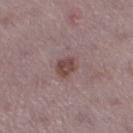| field | value |
|---|---|
| workup | no biopsy performed (imaged during a skin exam) |
| patient | female, aged approximately 50 |
| tile lighting | white-light illumination |
| acquisition | ~15 mm tile from a whole-body skin photo |
| lesion size | ~3 mm (longest diameter) |
| site | the left lower leg |
| automated metrics | a shape eccentricity near 0.65 and two-axis asymmetry of about 0.2; a lesion color around L≈46 a*≈18 b*≈20 in CIELAB and a lesion–skin lightness drop of about 9; a within-lesion color-variation index near 2/10 and a peripheral color-asymmetry measure near 0.5; a nevus-likeness score of about 75/100 |